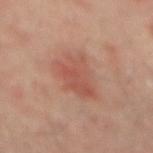Recorded during total-body skin imaging; not selected for excision or biopsy.
From the back.
Automated image analysis of the tile measured a lesion color around L≈51 a*≈24 b*≈28 in CIELAB, roughly 7 lightness units darker than nearby skin, and a lesion-to-skin contrast of about 5.5 (normalized; higher = more distinct).
A male patient aged approximately 65.
A 15 mm close-up extracted from a 3D total-body photography capture.
The tile uses cross-polarized illumination.
The recorded lesion diameter is about 5 mm.